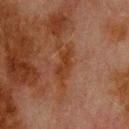<case>
  <biopsy_status>not biopsied; imaged during a skin examination</biopsy_status>
  <site>upper back</site>
  <patient>
    <sex>male</sex>
    <age_approx>80</age_approx>
  </patient>
  <image>
    <source>total-body photography crop</source>
    <field_of_view_mm>15</field_of_view_mm>
  </image>
</case>